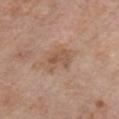Assessment:
The lesion was tiled from a total-body skin photograph and was not biopsied.
Context:
The lesion is located on the chest. The lesion-visualizer software estimated an area of roughly 6 mm² and an outline eccentricity of about 0.75 (0 = round, 1 = elongated). The software also gave a lesion color around L≈53 a*≈19 b*≈30 in CIELAB and about 8 CIELAB-L* units darker than the surrounding skin. And it measured a classifier nevus-likeness of about 0/100 and lesion-presence confidence of about 100/100. Cropped from a total-body skin-imaging series; the visible field is about 15 mm. Longest diameter approximately 3 mm. The subject is a male in their mid-60s.